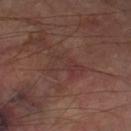Case summary:
* workup — total-body-photography surveillance lesion; no biopsy
* tile lighting — cross-polarized illumination
* diameter — ~4 mm (longest diameter)
* location — the leg
* patient — male, roughly 70 years of age
* imaging modality — ~15 mm crop, total-body skin-cancer survey